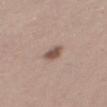<tbp_lesion>
<biopsy_status>not biopsied; imaged during a skin examination</biopsy_status>
<patient>
  <sex>female</sex>
  <age_approx>25</age_approx>
</patient>
<site>right thigh</site>
<image>
  <source>total-body photography crop</source>
  <field_of_view_mm>15</field_of_view_mm>
</image>
<lesion_size>
  <long_diameter_mm_approx>3.0</long_diameter_mm_approx>
</lesion_size>
<lighting>white-light</lighting>
</tbp_lesion>